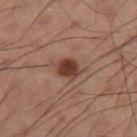This lesion was catalogued during total-body skin photography and was not selected for biopsy.
An algorithmic analysis of the crop reported a lesion color around L≈32 a*≈20 b*≈23 in CIELAB, a lesion–skin lightness drop of about 12, and a normalized lesion–skin contrast near 11. The analysis additionally found a border-irregularity rating of about 2/10, a color-variation rating of about 2.5/10, and radial color variation of about 0.5. And it measured a nevus-likeness score of about 95/100 and a lesion-detection confidence of about 100/100.
The tile uses cross-polarized illumination.
Longest diameter approximately 2.5 mm.
A male patient approximately 60 years of age.
A roughly 15 mm field-of-view crop from a total-body skin photograph.